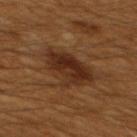Q: Was this lesion biopsied?
A: catalogued during a skin exam; not biopsied
Q: How was this image acquired?
A: total-body-photography crop, ~15 mm field of view
Q: Where on the body is the lesion?
A: the mid back
Q: What are the patient's age and sex?
A: male, approximately 60 years of age
Q: How large is the lesion?
A: about 5.5 mm
Q: Illumination type?
A: cross-polarized illumination